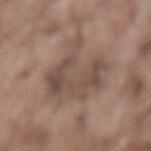Recorded during total-body skin imaging; not selected for excision or biopsy. Located on the lower back. This image is a 15 mm lesion crop taken from a total-body photograph. A male subject, approximately 75 years of age. This is a white-light tile.